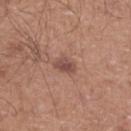Clinical impression: The lesion was photographed on a routine skin check and not biopsied; there is no pathology result. Clinical summary: The subject is a male aged 48–52. Located on the left lower leg. A roughly 15 mm field-of-view crop from a total-body skin photograph. The lesion's longest dimension is about 2.5 mm.